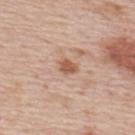workup: imaged on a skin check; not biopsied | illumination: white-light illumination | lesion diameter: ≈2.5 mm | image source: 15 mm crop, total-body photography | body site: the upper back | patient: male, in their mid-70s.